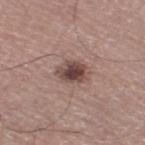Impression: The lesion was tiled from a total-body skin photograph and was not biopsied. Acquisition and patient details: The tile uses white-light illumination. From the right thigh. A male subject aged approximately 75. Longest diameter approximately 3.5 mm. A 15 mm close-up tile from a total-body photography series done for melanoma screening. Automated tile analysis of the lesion measured an area of roughly 7 mm², a shape eccentricity near 0.75, and a shape-asymmetry score of about 0.2 (0 = symmetric). It also reported an average lesion color of about L≈45 a*≈18 b*≈21 (CIELAB) and a normalized border contrast of about 10. The analysis additionally found a classifier nevus-likeness of about 85/100.